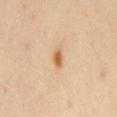patient: male, roughly 55 years of age; location: the chest; diameter: about 2.5 mm; acquisition: total-body-photography crop, ~15 mm field of view; illumination: cross-polarized.